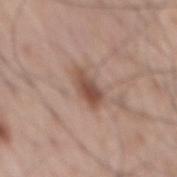Assessment:
This lesion was catalogued during total-body skin photography and was not selected for biopsy.
Background:
A 15 mm close-up extracted from a 3D total-body photography capture. An algorithmic analysis of the crop reported a shape eccentricity near 0.9 and two-axis asymmetry of about 0.2. A male subject, aged around 70. Captured under white-light illumination. About 4 mm across. The lesion is on the mid back.